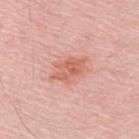Q: Was this lesion biopsied?
A: no biopsy performed (imaged during a skin exam)
Q: What kind of image is this?
A: 15 mm crop, total-body photography
Q: Lesion location?
A: the upper back
Q: Patient demographics?
A: male, aged 48–52
Q: What lighting was used for the tile?
A: white-light